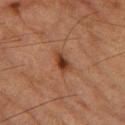This lesion was catalogued during total-body skin photography and was not selected for biopsy. Cropped from a whole-body photographic skin survey; the tile spans about 15 mm. A male subject in their mid-80s or older. On the leg. Automated tile analysis of the lesion measured an area of roughly 4 mm², an outline eccentricity of about 0.85 (0 = round, 1 = elongated), and a symmetry-axis asymmetry near 0.25. And it measured an average lesion color of about L≈34 a*≈21 b*≈29 (CIELAB). The analysis additionally found a border-irregularity index near 2.5/10, a color-variation rating of about 5/10, and a peripheral color-asymmetry measure near 1.5.